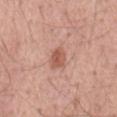Clinical summary: The subject is a male in their mid- to late 50s. The lesion is on the mid back. A lesion tile, about 15 mm wide, cut from a 3D total-body photograph.